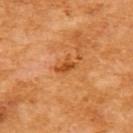The lesion was photographed on a routine skin check and not biopsied; there is no pathology result. The tile uses cross-polarized illumination. A lesion tile, about 15 mm wide, cut from a 3D total-body photograph. The recorded lesion diameter is about 2.5 mm. From the upper back. A female subject, aged approximately 55.